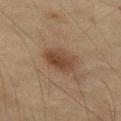follow-up=imaged on a skin check; not biopsied | patient=female, aged 53 to 57 | automated metrics=a lesion color around L≈38 a*≈16 b*≈26 in CIELAB, roughly 9 lightness units darker than nearby skin, and a normalized lesion–skin contrast near 8; a border-irregularity index near 2/10, a color-variation rating of about 3.5/10, and radial color variation of about 1 | image=total-body-photography crop, ~15 mm field of view | site=the left thigh | diameter=about 4 mm | lighting=cross-polarized.